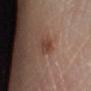Clinical impression: Captured during whole-body skin photography for melanoma surveillance; the lesion was not biopsied. Context: This image is a 15 mm lesion crop taken from a total-body photograph. The lesion's longest dimension is about 2.5 mm. From the right lower leg. The patient is a female in their 70s. Captured under white-light illumination.